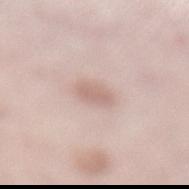The lesion was tiled from a total-body skin photograph and was not biopsied. A close-up tile cropped from a whole-body skin photograph, about 15 mm across. The lesion is on the leg. The lesion's longest dimension is about 2.5 mm. Imaged with white-light lighting. The subject is a female aged around 65. Automated tile analysis of the lesion measured an average lesion color of about L≈65 a*≈18 b*≈23 (CIELAB), about 9 CIELAB-L* units darker than the surrounding skin, and a normalized border contrast of about 5.5. The software also gave border irregularity of about 2 on a 0–10 scale, a color-variation rating of about 1/10, and radial color variation of about 0.5. It also reported a nevus-likeness score of about 85/100 and lesion-presence confidence of about 100/100.